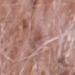{"biopsy_status": "not biopsied; imaged during a skin examination", "lighting": "white-light", "site": "right forearm", "lesion_size": {"long_diameter_mm_approx": 3.0}, "image": {"source": "total-body photography crop", "field_of_view_mm": 15}, "patient": {"sex": "male", "age_approx": 75}}